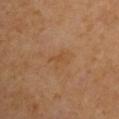Notes:
• lesion size · ~2.5 mm (longest diameter)
• image · ~15 mm crop, total-body skin-cancer survey
• patient · male, aged 63–67
• location · the left arm
• illumination · cross-polarized
• automated metrics · internal color variation of about 0.5 on a 0–10 scale and a peripheral color-asymmetry measure near 0